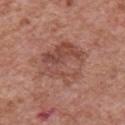Q: Was this lesion biopsied?
A: no biopsy performed (imaged during a skin exam)
Q: What is the lesion's diameter?
A: about 5.5 mm
Q: Illumination type?
A: white-light illumination
Q: How was this image acquired?
A: ~15 mm tile from a whole-body skin photo
Q: Who is the patient?
A: male, aged around 70
Q: What is the anatomic site?
A: the chest
Q: Automated lesion metrics?
A: roughly 8 lightness units darker than nearby skin and a normalized lesion–skin contrast near 6